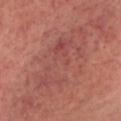Captured during whole-body skin photography for melanoma surveillance; the lesion was not biopsied.
This is a cross-polarized tile.
A male patient, in their 50s.
The lesion is on the head or neck.
The recorded lesion diameter is about 10 mm.
Cropped from a total-body skin-imaging series; the visible field is about 15 mm.